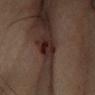No biopsy was performed on this lesion — it was imaged during a full skin examination and was not determined to be concerning.
The patient is a male aged approximately 50.
The tile uses cross-polarized illumination.
The lesion is located on the right forearm.
The lesion-visualizer software estimated a peripheral color-asymmetry measure near 2. The analysis additionally found a classifier nevus-likeness of about 80/100 and a lesion-detection confidence of about 80/100.
A region of skin cropped from a whole-body photographic capture, roughly 15 mm wide.